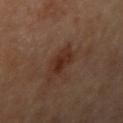Findings:
* follow-up: imaged on a skin check; not biopsied
* imaging modality: ~15 mm tile from a whole-body skin photo
* location: the right upper arm
* patient: male, aged 83 or over
* diameter: ~3.5 mm (longest diameter)
* lighting: cross-polarized illumination